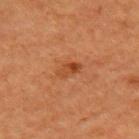Clinical impression: Part of a total-body skin-imaging series; this lesion was reviewed on a skin check and was not flagged for biopsy. Clinical summary: Located on the arm. Automated image analysis of the tile measured a lesion area of about 3.5 mm², an eccentricity of roughly 0.85, and a symmetry-axis asymmetry near 0.3. It also reported an average lesion color of about L≈39 a*≈25 b*≈35 (CIELAB) and a lesion–skin lightness drop of about 8. The software also gave a border-irregularity index near 3/10, a within-lesion color-variation index near 4.5/10, and radial color variation of about 1.5. It also reported lesion-presence confidence of about 100/100. The recorded lesion diameter is about 3 mm. A close-up tile cropped from a whole-body skin photograph, about 15 mm across. A female patient aged approximately 55. Captured under cross-polarized illumination.